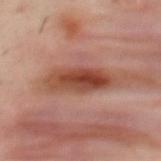Impression:
Captured during whole-body skin photography for melanoma surveillance; the lesion was not biopsied.
Background:
A male subject aged approximately 40. From the upper back. This is a cross-polarized tile. A lesion tile, about 15 mm wide, cut from a 3D total-body photograph. Approximately 7 mm at its widest.